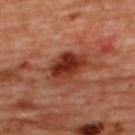biopsy status = total-body-photography surveillance lesion; no biopsy
image = total-body-photography crop, ~15 mm field of view
lighting = cross-polarized illumination
automated metrics = an area of roughly 12 mm², an eccentricity of roughly 0.7, and a symmetry-axis asymmetry near 0.25; border irregularity of about 2.5 on a 0–10 scale and peripheral color asymmetry of about 2.5; an automated nevus-likeness rating near 85 out of 100 and a detector confidence of about 100 out of 100 that the crop contains a lesion
body site = the upper back
subject = female, about 45 years old